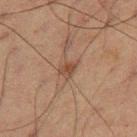workup = catalogued during a skin exam; not biopsied
acquisition = 15 mm crop, total-body photography
subject = male, roughly 60 years of age
location = the right thigh